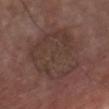<lesion>
<biopsy_status>not biopsied; imaged during a skin examination</biopsy_status>
<patient>
  <sex>male</sex>
  <age_approx>80</age_approx>
</patient>
<image>
  <source>total-body photography crop</source>
  <field_of_view_mm>15</field_of_view_mm>
</image>
<lesion_size>
  <long_diameter_mm_approx>7.5</long_diameter_mm_approx>
</lesion_size>
<site>chest</site>
</lesion>